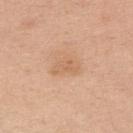image:
  source: total-body photography crop
  field_of_view_mm: 15
site: right upper arm
patient:
  sex: female
  age_approx: 40
lighting: white-light
lesion_size:
  long_diameter_mm_approx: 3.5